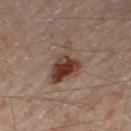Clinical impression: The lesion was photographed on a routine skin check and not biopsied; there is no pathology result. Clinical summary: A 15 mm close-up extracted from a 3D total-body photography capture. This is a cross-polarized tile. About 5.5 mm across. The lesion is located on the right thigh. An algorithmic analysis of the crop reported a lesion color around L≈33 a*≈17 b*≈21 in CIELAB, roughly 11 lightness units darker than nearby skin, and a normalized border contrast of about 10.5. And it measured a border-irregularity index near 5.5/10, a color-variation rating of about 6.5/10, and radial color variation of about 2. A male patient, aged approximately 45.